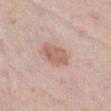– follow-up — imaged on a skin check; not biopsied
– diameter — about 4 mm
– TBP lesion metrics — an automated nevus-likeness rating near 90 out of 100 and lesion-presence confidence of about 100/100
– subject — female, in their mid-40s
– anatomic site — the leg
– tile lighting — white-light illumination
– acquisition — 15 mm crop, total-body photography